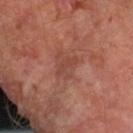{"biopsy_status": "not biopsied; imaged during a skin examination", "site": "arm", "image": {"source": "total-body photography crop", "field_of_view_mm": 15}, "lighting": "cross-polarized", "patient": {"sex": "male", "age_approx": 70}}